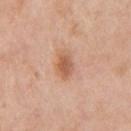workup: catalogued during a skin exam; not biopsied
diameter: ~3 mm (longest diameter)
subject: female, approximately 40 years of age
body site: the arm
lighting: white-light illumination
image-analysis metrics: a symmetry-axis asymmetry near 0.15; a border-irregularity rating of about 1.5/10, internal color variation of about 3.5 on a 0–10 scale, and a peripheral color-asymmetry measure near 1; an automated nevus-likeness rating near 85 out of 100 and lesion-presence confidence of about 100/100
acquisition: ~15 mm tile from a whole-body skin photo